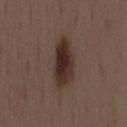Context:
The recorded lesion diameter is about 6.5 mm. A male patient approximately 55 years of age. On the mid back. This image is a 15 mm lesion crop taken from a total-body photograph.
Conclusion:
Histopathology of the biopsied lesion showed a dysplastic (Clark) nevus.